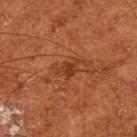This lesion was catalogued during total-body skin photography and was not selected for biopsy.
A roughly 15 mm field-of-view crop from a total-body skin photograph.
A male patient, about 65 years old.
On the left lower leg.
Approximately 3 mm at its widest.
An algorithmic analysis of the crop reported an average lesion color of about L≈36 a*≈27 b*≈34 (CIELAB) and a lesion-to-skin contrast of about 7 (normalized; higher = more distinct). It also reported border irregularity of about 4.5 on a 0–10 scale, internal color variation of about 1.5 on a 0–10 scale, and peripheral color asymmetry of about 0.5.